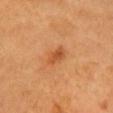Part of a total-body skin-imaging series; this lesion was reviewed on a skin check and was not flagged for biopsy.
About 3 mm across.
This is a cross-polarized tile.
A 15 mm crop from a total-body photograph taken for skin-cancer surveillance.
Located on the chest.
A female patient aged 63 to 67.